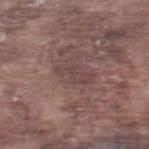Impression: Part of a total-body skin-imaging series; this lesion was reviewed on a skin check and was not flagged for biopsy.